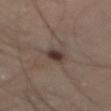| feature | finding |
|---|---|
| biopsy status | total-body-photography surveillance lesion; no biopsy |
| imaging modality | ~15 mm crop, total-body skin-cancer survey |
| lesion size | about 3 mm |
| anatomic site | the front of the torso |
| tile lighting | cross-polarized |
| image-analysis metrics | a lesion area of about 4 mm², an outline eccentricity of about 0.8 (0 = round, 1 = elongated), and two-axis asymmetry of about 0.25; a border-irregularity rating of about 2.5/10 and internal color variation of about 3 on a 0–10 scale |
| patient | male, aged 63 to 67 |